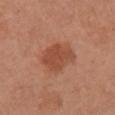| field | value |
|---|---|
| biopsy status | catalogued during a skin exam; not biopsied |
| subject | female, aged 63–67 |
| image | ~15 mm tile from a whole-body skin photo |
| illumination | white-light |
| image-analysis metrics | an area of roughly 12 mm², an outline eccentricity of about 0.6 (0 = round, 1 = elongated), and a shape-asymmetry score of about 0.2 (0 = symmetric); a lesion color around L≈49 a*≈26 b*≈33 in CIELAB and a lesion–skin lightness drop of about 9; a classifier nevus-likeness of about 80/100 and a detector confidence of about 100 out of 100 that the crop contains a lesion |
| anatomic site | the chest |